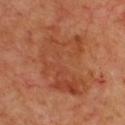  site: chest
  patient:
    sex: male
    age_approx: 65
  image:
    source: total-body photography crop
    field_of_view_mm: 15